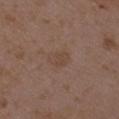workup = no biopsy performed (imaged during a skin exam)
lesion size = ~3 mm (longest diameter)
anatomic site = the arm
imaging modality = ~15 mm crop, total-body skin-cancer survey
patient = female, in their mid-30s